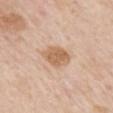Imaged during a routine full-body skin examination; the lesion was not biopsied and no histopathology is available. A 15 mm crop from a total-body photograph taken for skin-cancer surveillance. The recorded lesion diameter is about 4 mm. Located on the mid back. The subject is a male in their mid-80s or older. Captured under white-light illumination.